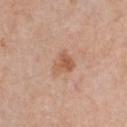Q: Was a biopsy performed?
A: catalogued during a skin exam; not biopsied
Q: Who is the patient?
A: male, aged 58 to 62
Q: What lighting was used for the tile?
A: white-light illumination
Q: What kind of image is this?
A: total-body-photography crop, ~15 mm field of view
Q: Automated lesion metrics?
A: a lesion area of about 5.5 mm², an outline eccentricity of about 0.5 (0 = round, 1 = elongated), and two-axis asymmetry of about 0.35; a within-lesion color-variation index near 3.5/10 and a peripheral color-asymmetry measure near 1; an automated nevus-likeness rating near 35 out of 100 and a lesion-detection confidence of about 100/100
Q: What is the lesion's diameter?
A: ~3 mm (longest diameter)
Q: Where on the body is the lesion?
A: the chest